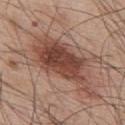The lesion was tiled from a total-body skin photograph and was not biopsied.
Captured under white-light illumination.
The subject is a male aged 53–57.
Measured at roughly 9.5 mm in maximum diameter.
A 15 mm close-up extracted from a 3D total-body photography capture.
On the upper back.